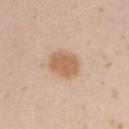biopsy status: total-body-photography surveillance lesion; no biopsy | automated lesion analysis: roughly 11 lightness units darker than nearby skin and a lesion-to-skin contrast of about 7.5 (normalized; higher = more distinct) | tile lighting: white-light | size: ~3.5 mm (longest diameter) | acquisition: 15 mm crop, total-body photography | anatomic site: the arm | patient: female, aged 18 to 22.